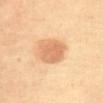Findings:
- workup: no biopsy performed (imaged during a skin exam)
- TBP lesion metrics: a mean CIELAB color near L≈71 a*≈24 b*≈40 and a lesion–skin lightness drop of about 12
- imaging modality: ~15 mm tile from a whole-body skin photo
- tile lighting: cross-polarized illumination
- site: the abdomen
- patient: female, roughly 65 years of age
- diameter: ~4 mm (longest diameter)